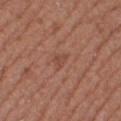Part of a total-body skin-imaging series; this lesion was reviewed on a skin check and was not flagged for biopsy.
The lesion is located on the right thigh.
Measured at roughly 2.5 mm in maximum diameter.
Automated image analysis of the tile measured a lesion area of about 3 mm², a shape eccentricity near 0.8, and a symmetry-axis asymmetry near 0.3. It also reported a lesion–skin lightness drop of about 6. And it measured an automated nevus-likeness rating near 0 out of 100 and a lesion-detection confidence of about 100/100.
Cropped from a total-body skin-imaging series; the visible field is about 15 mm.
The subject is a female approximately 40 years of age.